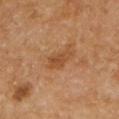biopsy_status: not biopsied; imaged during a skin examination
lighting: cross-polarized
site: left upper arm
image:
  source: total-body photography crop
  field_of_view_mm: 15
patient:
  sex: male
  age_approx: 55
automated_metrics:
  eccentricity: 0.85
  shape_asymmetry: 0.25
  cielab_L: 45
  cielab_a: 21
  cielab_b: 35
  vs_skin_darker_L: 7.0
  vs_skin_contrast_norm: 6.5